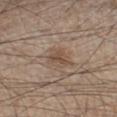Part of a total-body skin-imaging series; this lesion was reviewed on a skin check and was not flagged for biopsy.
About 3 mm across.
This is a white-light tile.
A roughly 15 mm field-of-view crop from a total-body skin photograph.
A male patient, about 45 years old.
Located on the left lower leg.